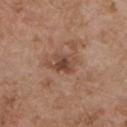No biopsy was performed on this lesion — it was imaged during a full skin examination and was not determined to be concerning. A 15 mm close-up extracted from a 3D total-body photography capture. Longest diameter approximately 3.5 mm. The lesion is located on the chest. A male patient roughly 55 years of age. Automated tile analysis of the lesion measured a lesion area of about 6 mm², an outline eccentricity of about 0.65 (0 = round, 1 = elongated), and two-axis asymmetry of about 0.3. And it measured a mean CIELAB color near L≈45 a*≈20 b*≈28 and a normalized border contrast of about 8. The analysis additionally found internal color variation of about 4.5 on a 0–10 scale and radial color variation of about 1.5.